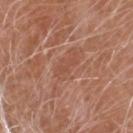Assessment: Part of a total-body skin-imaging series; this lesion was reviewed on a skin check and was not flagged for biopsy. Image and clinical context: The tile uses white-light illumination. On the head or neck. A male subject aged approximately 55. Automated image analysis of the tile measured a lesion area of about 3 mm² and a shape eccentricity near 0.95. It also reported a mean CIELAB color near L≈50 a*≈24 b*≈31, roughly 6 lightness units darker than nearby skin, and a normalized lesion–skin contrast near 4.5. Cropped from a total-body skin-imaging series; the visible field is about 15 mm.